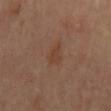Q: Is there a histopathology result?
A: catalogued during a skin exam; not biopsied
Q: Automated lesion metrics?
A: a lesion area of about 3.5 mm², an eccentricity of roughly 0.8, and two-axis asymmetry of about 0.35; an average lesion color of about L≈41 a*≈20 b*≈29 (CIELAB) and a lesion-to-skin contrast of about 5.5 (normalized; higher = more distinct); a within-lesion color-variation index near 1/10
Q: Where on the body is the lesion?
A: the back
Q: How was the tile lit?
A: cross-polarized
Q: How was this image acquired?
A: ~15 mm tile from a whole-body skin photo
Q: What is the lesion's diameter?
A: ≈2.5 mm